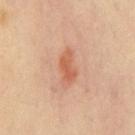  biopsy_status: not biopsied; imaged during a skin examination
  automated_metrics:
    shape_asymmetry: 0.3
    cielab_L: 58
    cielab_a: 25
    cielab_b: 34
    vs_skin_darker_L: 9.0
    vs_skin_contrast_norm: 7.0
    nevus_likeness_0_100: 80
    lesion_detection_confidence_0_100: 100
  site: chest
  image:
    source: total-body photography crop
    field_of_view_mm: 15
  patient:
    sex: male
    age_approx: 50